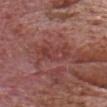<lesion>
  <biopsy_status>not biopsied; imaged during a skin examination</biopsy_status>
  <lighting>white-light</lighting>
  <image>
    <source>total-body photography crop</source>
    <field_of_view_mm>15</field_of_view_mm>
  </image>
  <site>head or neck</site>
  <automated_metrics>
    <vs_skin_contrast_norm>5.0</vs_skin_contrast_norm>
  </automated_metrics>
  <lesion_size>
    <long_diameter_mm_approx>4.5</long_diameter_mm_approx>
  </lesion_size>
  <patient>
    <sex>male</sex>
    <age_approx>75</age_approx>
  </patient>
</lesion>